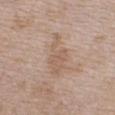Imaged during a routine full-body skin examination; the lesion was not biopsied and no histopathology is available. A male patient, in their 50s. A region of skin cropped from a whole-body photographic capture, roughly 15 mm wide. The lesion is located on the chest. The lesion's longest dimension is about 6 mm. The total-body-photography lesion software estimated a footprint of about 10 mm², an eccentricity of roughly 0.9, and a shape-asymmetry score of about 0.35 (0 = symmetric). Imaged with white-light lighting.